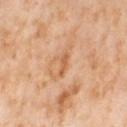follow-up: imaged on a skin check; not biopsied
lighting: cross-polarized
image: ~15 mm tile from a whole-body skin photo
automated lesion analysis: a footprint of about 2.5 mm², an outline eccentricity of about 0.9 (0 = round, 1 = elongated), and a shape-asymmetry score of about 0.3 (0 = symmetric); lesion-presence confidence of about 100/100
subject: female, aged approximately 55
lesion diameter: ≈2.5 mm
anatomic site: the right thigh